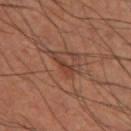Q: Is there a histopathology result?
A: total-body-photography surveillance lesion; no biopsy
Q: Who is the patient?
A: male, aged approximately 60
Q: Automated lesion metrics?
A: a border-irregularity rating of about 6.5/10 and a within-lesion color-variation index near 1/10; a nevus-likeness score of about 0/100 and a lesion-detection confidence of about 55/100
Q: What is the imaging modality?
A: 15 mm crop, total-body photography
Q: Where on the body is the lesion?
A: the left thigh
Q: What lighting was used for the tile?
A: cross-polarized illumination